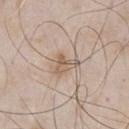Imaged during a routine full-body skin examination; the lesion was not biopsied and no histopathology is available. The subject is a male in their mid- to late 50s. A roughly 15 mm field-of-view crop from a total-body skin photograph. From the front of the torso. An algorithmic analysis of the crop reported a lesion–skin lightness drop of about 9. The lesion's longest dimension is about 3 mm.